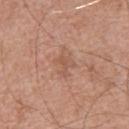No biopsy was performed on this lesion — it was imaged during a full skin examination and was not determined to be concerning.
From the upper back.
The subject is a male aged approximately 65.
Cropped from a whole-body photographic skin survey; the tile spans about 15 mm.